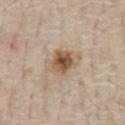workup = no biopsy performed (imaged during a skin exam)
acquisition = ~15 mm crop, total-body skin-cancer survey
size = ~4.5 mm (longest diameter)
lighting = white-light illumination
subject = male, in their 70s
TBP lesion metrics = an area of roughly 9.5 mm² and a symmetry-axis asymmetry near 0.25; about 12 CIELAB-L* units darker than the surrounding skin and a normalized border contrast of about 9; a border-irregularity rating of about 3/10 and a color-variation rating of about 7/10; a nevus-likeness score of about 90/100 and a detector confidence of about 100 out of 100 that the crop contains a lesion
anatomic site = the front of the torso